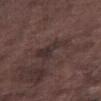This lesion was catalogued during total-body skin photography and was not selected for biopsy.
Longest diameter approximately 4.5 mm.
On the right thigh.
A male patient approximately 75 years of age.
A lesion tile, about 15 mm wide, cut from a 3D total-body photograph.
This is a white-light tile.
An algorithmic analysis of the crop reported an area of roughly 7 mm² and an outline eccentricity of about 0.9 (0 = round, 1 = elongated). The software also gave a border-irregularity index near 6.5/10 and a color-variation rating of about 2.5/10.